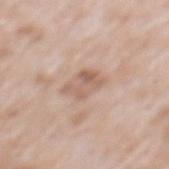{
  "biopsy_status": "not biopsied; imaged during a skin examination",
  "lesion_size": {
    "long_diameter_mm_approx": 3.5
  },
  "patient": {
    "sex": "male",
    "age_approx": 50
  },
  "automated_metrics": {
    "area_mm2_approx": 7.5,
    "eccentricity": 0.7,
    "shape_asymmetry": 0.25,
    "nevus_likeness_0_100": 0,
    "lesion_detection_confidence_0_100": 100
  },
  "site": "mid back",
  "image": {
    "source": "total-body photography crop",
    "field_of_view_mm": 15
  }
}